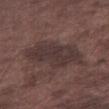Impression: The lesion was tiled from a total-body skin photograph and was not biopsied. Image and clinical context: A 15 mm close-up extracted from a 3D total-body photography capture. The lesion is located on the right thigh. A male subject aged around 75.